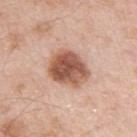{
  "biopsy_status": "not biopsied; imaged during a skin examination",
  "automated_metrics": {
    "area_mm2_approx": 15.0,
    "eccentricity": 0.6,
    "shape_asymmetry": 0.15,
    "border_irregularity_0_10": 1.5,
    "color_variation_0_10": 6.0,
    "peripheral_color_asymmetry": 2.0,
    "nevus_likeness_0_100": 60,
    "lesion_detection_confidence_0_100": 100
  },
  "image": {
    "source": "total-body photography crop",
    "field_of_view_mm": 15
  },
  "patient": {
    "sex": "male",
    "age_approx": 55
  },
  "site": "left upper arm",
  "lighting": "white-light"
}